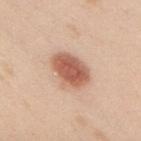follow-up: no biopsy performed (imaged during a skin exam).